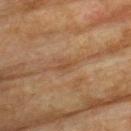Background: A male patient, roughly 75 years of age. A 15 mm crop from a total-body photograph taken for skin-cancer surveillance. The lesion is located on the back. Measured at roughly 2.5 mm in maximum diameter.